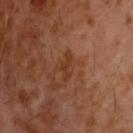Assessment: This lesion was catalogued during total-body skin photography and was not selected for biopsy. Background: This image is a 15 mm lesion crop taken from a total-body photograph. The recorded lesion diameter is about 3.5 mm. From the head or neck. A male subject, aged 58 to 62.